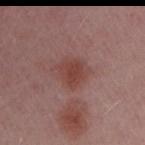| key | value |
|---|---|
| biopsy status | catalogued during a skin exam; not biopsied |
| image-analysis metrics | a mean CIELAB color near L≈42 a*≈24 b*≈23, a lesion–skin lightness drop of about 8, and a lesion-to-skin contrast of about 7 (normalized; higher = more distinct); border irregularity of about 2.5 on a 0–10 scale, a within-lesion color-variation index near 2/10, and radial color variation of about 0.5 |
| subject | female, about 45 years old |
| site | the right upper arm |
| lighting | white-light |
| acquisition | 15 mm crop, total-body photography |
| size | about 3 mm |